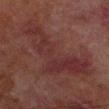Q: Was this lesion biopsied?
A: imaged on a skin check; not biopsied
Q: What kind of image is this?
A: ~15 mm crop, total-body skin-cancer survey
Q: Who is the patient?
A: male, about 70 years old
Q: What lighting was used for the tile?
A: cross-polarized
Q: How large is the lesion?
A: about 11 mm
Q: Where on the body is the lesion?
A: the left lower leg